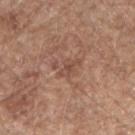Q: Was this lesion biopsied?
A: imaged on a skin check; not biopsied
Q: What is the anatomic site?
A: the right forearm
Q: How large is the lesion?
A: ~3.5 mm (longest diameter)
Q: Illumination type?
A: white-light illumination
Q: What are the patient's age and sex?
A: male, aged around 60
Q: Automated lesion metrics?
A: an average lesion color of about L≈49 a*≈20 b*≈27 (CIELAB), about 7 CIELAB-L* units darker than the surrounding skin, and a normalized border contrast of about 5.5; lesion-presence confidence of about 100/100
Q: What kind of image is this?
A: 15 mm crop, total-body photography